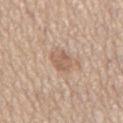Case summary:
- workup — catalogued during a skin exam; not biopsied
- subject — male, about 65 years old
- imaging modality — 15 mm crop, total-body photography
- illumination — white-light illumination
- size — about 3 mm
- automated metrics — an automated nevus-likeness rating near 15 out of 100 and lesion-presence confidence of about 100/100
- site — the chest